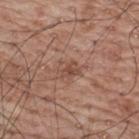Case summary:
– location · the upper back
– automated lesion analysis · a mean CIELAB color near L≈49 a*≈21 b*≈27, a lesion–skin lightness drop of about 8, and a normalized lesion–skin contrast near 6; border irregularity of about 3 on a 0–10 scale, internal color variation of about 2 on a 0–10 scale, and a peripheral color-asymmetry measure near 0.5; a detector confidence of about 100 out of 100 that the crop contains a lesion
– size · ~2.5 mm (longest diameter)
– lighting · white-light
– acquisition · 15 mm crop, total-body photography
– patient · male, about 70 years old